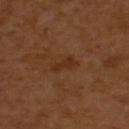| feature | finding |
|---|---|
| notes | imaged on a skin check; not biopsied |
| lesion size | ≈2.5 mm |
| anatomic site | the right upper arm |
| image source | 15 mm crop, total-body photography |
| TBP lesion metrics | a lesion color around L≈31 a*≈20 b*≈32 in CIELAB, a lesion–skin lightness drop of about 6, and a normalized border contrast of about 6.5; a border-irregularity rating of about 3.5/10, a within-lesion color-variation index near 0.5/10, and peripheral color asymmetry of about 0 |
| subject | female, roughly 55 years of age |
| illumination | cross-polarized |